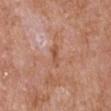{"biopsy_status": "not biopsied; imaged during a skin examination", "image": {"source": "total-body photography crop", "field_of_view_mm": 15}, "patient": {"sex": "male", "age_approx": 65}, "lighting": "white-light", "site": "right upper arm", "automated_metrics": {"eccentricity": 0.9, "cielab_L": 54, "cielab_a": 24, "cielab_b": 32, "vs_skin_darker_L": 8.0, "vs_skin_contrast_norm": 6.5, "border_irregularity_0_10": 2.5, "color_variation_0_10": 2.0, "peripheral_color_asymmetry": 0.0, "lesion_detection_confidence_0_100": 100}, "lesion_size": {"long_diameter_mm_approx": 3.0}}